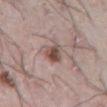Q: Was a biopsy performed?
A: imaged on a skin check; not biopsied
Q: Automated lesion metrics?
A: a lesion area of about 5.5 mm² and an eccentricity of roughly 0.6; an average lesion color of about L≈50 a*≈18 b*≈22 (CIELAB); border irregularity of about 2 on a 0–10 scale and radial color variation of about 2; a nevus-likeness score of about 85/100 and a detector confidence of about 100 out of 100 that the crop contains a lesion
Q: What is the anatomic site?
A: the abdomen
Q: What kind of image is this?
A: total-body-photography crop, ~15 mm field of view
Q: How large is the lesion?
A: ≈3 mm
Q: What lighting was used for the tile?
A: white-light illumination
Q: Patient demographics?
A: male, about 75 years old